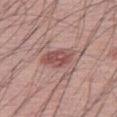Clinical impression:
The lesion was tiled from a total-body skin photograph and was not biopsied.
Clinical summary:
A roughly 15 mm field-of-view crop from a total-body skin photograph. Approximately 4 mm at its widest. A male subject aged approximately 55. Located on the right thigh. Automated image analysis of the tile measured roughly 10 lightness units darker than nearby skin.